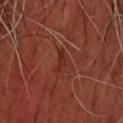Impression: Imaged during a routine full-body skin examination; the lesion was not biopsied and no histopathology is available. Clinical summary: Imaged with cross-polarized lighting. A lesion tile, about 15 mm wide, cut from a 3D total-body photograph. Measured at roughly 3 mm in maximum diameter. Located on the head or neck. A male patient about 45 years old. Automated image analysis of the tile measured a lesion area of about 4 mm², an outline eccentricity of about 0.85 (0 = round, 1 = elongated), and a symmetry-axis asymmetry near 0.4. It also reported an automated nevus-likeness rating near 0 out of 100 and lesion-presence confidence of about 60/100.